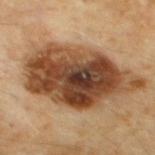Recorded during total-body skin imaging; not selected for excision or biopsy. Automated tile analysis of the lesion measured an area of roughly 55 mm², an eccentricity of roughly 0.85, and a symmetry-axis asymmetry near 0.2. The analysis additionally found a border-irregularity rating of about 3/10 and peripheral color asymmetry of about 3. A male subject, aged around 60. A 15 mm close-up tile from a total-body photography series done for melanoma screening. The lesion is on the chest.